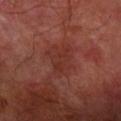The lesion was photographed on a routine skin check and not biopsied; there is no pathology result.
Located on the right forearm.
A region of skin cropped from a whole-body photographic capture, roughly 15 mm wide.
The total-body-photography lesion software estimated an area of roughly 6 mm². It also reported about 5 CIELAB-L* units darker than the surrounding skin and a normalized border contrast of about 5. And it measured a border-irregularity rating of about 4.5/10 and radial color variation of about 0.5.
Imaged with cross-polarized lighting.
The recorded lesion diameter is about 4 mm.
A male subject aged approximately 70.